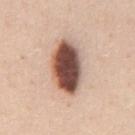biopsy status=imaged on a skin check; not biopsied
imaging modality=total-body-photography crop, ~15 mm field of view
automated lesion analysis=about 25 CIELAB-L* units darker than the surrounding skin; a peripheral color-asymmetry measure near 2.5
lesion diameter=about 6.5 mm
body site=the chest
subject=male, aged 58 to 62